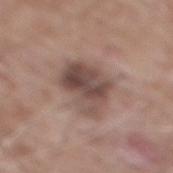{
  "biopsy_status": "not biopsied; imaged during a skin examination",
  "patient": {
    "sex": "male",
    "age_approx": 60
  },
  "image": {
    "source": "total-body photography crop",
    "field_of_view_mm": 15
  },
  "site": "mid back"
}